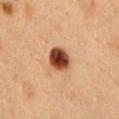Impression:
Part of a total-body skin-imaging series; this lesion was reviewed on a skin check and was not flagged for biopsy.
Background:
A male subject aged approximately 50. From the chest. This is a cross-polarized tile. Measured at roughly 3.5 mm in maximum diameter. Cropped from a total-body skin-imaging series; the visible field is about 15 mm.